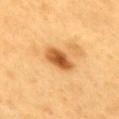Q: Is there a histopathology result?
A: catalogued during a skin exam; not biopsied
Q: Patient demographics?
A: male, aged 58 to 62
Q: Illumination type?
A: cross-polarized
Q: Where on the body is the lesion?
A: the mid back
Q: How was this image acquired?
A: ~15 mm crop, total-body skin-cancer survey
Q: What is the lesion's diameter?
A: about 4.5 mm